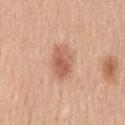Captured during whole-body skin photography for melanoma surveillance; the lesion was not biopsied. On the back. A lesion tile, about 15 mm wide, cut from a 3D total-body photograph. The patient is a male approximately 50 years of age.